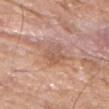<lesion>
  <biopsy_status>not biopsied; imaged during a skin examination</biopsy_status>
  <site>left forearm</site>
  <image>
    <source>total-body photography crop</source>
    <field_of_view_mm>15</field_of_view_mm>
  </image>
  <automated_metrics>
    <lesion_detection_confidence_0_100>100</lesion_detection_confidence_0_100>
  </automated_metrics>
  <lighting>white-light</lighting>
  <patient>
    <sex>male</sex>
    <age_approx>80</age_approx>
  </patient>
  <lesion_size>
    <long_diameter_mm_approx>4.0</long_diameter_mm_approx>
  </lesion_size>
</lesion>